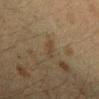site = the right forearm
imaging modality = total-body-photography crop, ~15 mm field of view
patient = female, roughly 40 years of age
lesion diameter = ≈3 mm
tile lighting = cross-polarized illumination